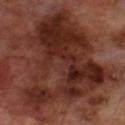Impression: No biopsy was performed on this lesion — it was imaged during a full skin examination and was not determined to be concerning. Background: The total-body-photography lesion software estimated a lesion area of about 70 mm², an outline eccentricity of about 0.8 (0 = round, 1 = elongated), and a shape-asymmetry score of about 0.55 (0 = symmetric). The software also gave a within-lesion color-variation index near 8/10. The lesion is on the right lower leg. The subject is a male aged 68–72. A roughly 15 mm field-of-view crop from a total-body skin photograph. This is a cross-polarized tile. Measured at roughly 14 mm in maximum diameter.